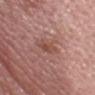Findings:
• biopsy status: total-body-photography surveillance lesion; no biopsy
• image: ~15 mm crop, total-body skin-cancer survey
• site: the head or neck
• patient: male, about 45 years old